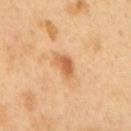| field | value |
|---|---|
| follow-up | imaged on a skin check; not biopsied |
| image source | 15 mm crop, total-body photography |
| subject | male, in their 50s |
| anatomic site | the upper back |
| diameter | ≈3 mm |
| illumination | cross-polarized illumination |